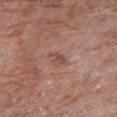Q: Is there a histopathology result?
A: total-body-photography surveillance lesion; no biopsy
Q: How was this image acquired?
A: 15 mm crop, total-body photography
Q: What are the patient's age and sex?
A: female, aged approximately 70
Q: Automated lesion metrics?
A: a lesion color around L≈49 a*≈22 b*≈26 in CIELAB, about 7 CIELAB-L* units darker than the surrounding skin, and a lesion-to-skin contrast of about 6 (normalized; higher = more distinct); a border-irregularity index near 4/10, internal color variation of about 1 on a 0–10 scale, and peripheral color asymmetry of about 0.5; a classifier nevus-likeness of about 0/100 and a detector confidence of about 100 out of 100 that the crop contains a lesion
Q: Lesion location?
A: the right upper arm
Q: Illumination type?
A: white-light illumination